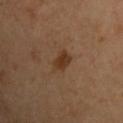Recorded during total-body skin imaging; not selected for excision or biopsy.